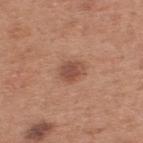Part of a total-body skin-imaging series; this lesion was reviewed on a skin check and was not flagged for biopsy. Located on the upper back. Captured under white-light illumination. About 3 mm across. A 15 mm close-up tile from a total-body photography series done for melanoma screening. A male patient, about 55 years old.